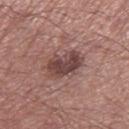Imaged during a routine full-body skin examination; the lesion was not biopsied and no histopathology is available.
A roughly 15 mm field-of-view crop from a total-body skin photograph.
A male subject, aged 68 to 72.
On the right thigh.
The tile uses white-light illumination.
Longest diameter approximately 4.5 mm.